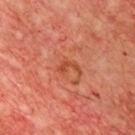notes = total-body-photography surveillance lesion; no biopsy
location = the chest
image-analysis metrics = a footprint of about 2.5 mm² and an eccentricity of roughly 0.85; roughly 8 lightness units darker than nearby skin; a border-irregularity index near 6.5/10 and a within-lesion color-variation index near 0/10
subject = male, aged approximately 60
image = total-body-photography crop, ~15 mm field of view
lighting = cross-polarized illumination